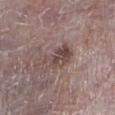Part of a total-body skin-imaging series; this lesion was reviewed on a skin check and was not flagged for biopsy.
The tile uses white-light illumination.
A roughly 15 mm field-of-view crop from a total-body skin photograph.
The recorded lesion diameter is about 4 mm.
Automated image analysis of the tile measured a lesion area of about 8 mm². It also reported a lesion color around L≈45 a*≈17 b*≈18 in CIELAB, roughly 9 lightness units darker than nearby skin, and a lesion-to-skin contrast of about 7 (normalized; higher = more distinct). The software also gave an automated nevus-likeness rating near 15 out of 100.
On the leg.
The subject is a male aged around 75.